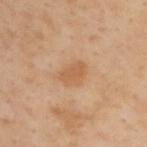Imaged during a routine full-body skin examination; the lesion was not biopsied and no histopathology is available.
Automated tile analysis of the lesion measured a footprint of about 5.5 mm² and a symmetry-axis asymmetry near 0.15. The analysis additionally found an average lesion color of about L≈59 a*≈21 b*≈37 (CIELAB).
A male subject, about 55 years old.
Captured under cross-polarized illumination.
From the upper back.
Cropped from a total-body skin-imaging series; the visible field is about 15 mm.
Approximately 3 mm at its widest.